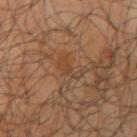Recorded during total-body skin imaging; not selected for excision or biopsy. This is a cross-polarized tile. Located on the right upper arm. Longest diameter approximately 3.5 mm. A close-up tile cropped from a whole-body skin photograph, about 15 mm across. A male patient aged 63–67.